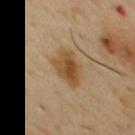* follow-up — imaged on a skin check; not biopsied
* patient — male, aged 48 to 52
* image source — ~15 mm crop, total-body skin-cancer survey
* diameter — ~4 mm (longest diameter)
* location — the chest